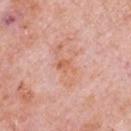Recorded during total-body skin imaging; not selected for excision or biopsy. A male patient, aged 78 to 82. A roughly 15 mm field-of-view crop from a total-body skin photograph. Longest diameter approximately 2.5 mm. The lesion is on the front of the torso.